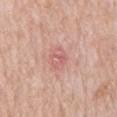| feature | finding |
|---|---|
| biopsy status | imaged on a skin check; not biopsied |
| TBP lesion metrics | a border-irregularity rating of about 2.5/10, internal color variation of about 3.5 on a 0–10 scale, and peripheral color asymmetry of about 1; a classifier nevus-likeness of about 0/100 and a detector confidence of about 100 out of 100 that the crop contains a lesion |
| site | the back |
| subject | male, aged 58–62 |
| tile lighting | white-light |
| image | 15 mm crop, total-body photography |